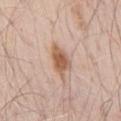<case>
  <biopsy_status>not biopsied; imaged during a skin examination</biopsy_status>
  <patient>
    <sex>male</sex>
    <age_approx>70</age_approx>
  </patient>
  <image>
    <source>total-body photography crop</source>
    <field_of_view_mm>15</field_of_view_mm>
  </image>
  <lesion_size>
    <long_diameter_mm_approx>4.0</long_diameter_mm_approx>
  </lesion_size>
  <site>right upper arm</site>
</case>